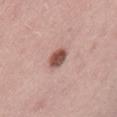workup — imaged on a skin check; not biopsied | illumination — white-light illumination | patient — male, in their mid-50s | location — the lower back | image — ~15 mm tile from a whole-body skin photo | size — about 2.5 mm | automated lesion analysis — a shape eccentricity near 0.65; a within-lesion color-variation index near 3/10 and peripheral color asymmetry of about 1; a nevus-likeness score of about 90/100 and a detector confidence of about 100 out of 100 that the crop contains a lesion.